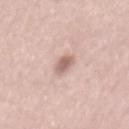Q: Was this lesion biopsied?
A: total-body-photography surveillance lesion; no biopsy
Q: Automated lesion metrics?
A: about 13 CIELAB-L* units darker than the surrounding skin; border irregularity of about 2 on a 0–10 scale, internal color variation of about 1.5 on a 0–10 scale, and radial color variation of about 0.5
Q: What is the imaging modality?
A: total-body-photography crop, ~15 mm field of view
Q: Illumination type?
A: white-light
Q: Where on the body is the lesion?
A: the mid back
Q: Who is the patient?
A: male, approximately 55 years of age